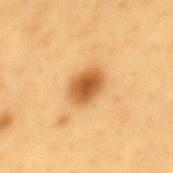Imaged during a routine full-body skin examination; the lesion was not biopsied and no histopathology is available.
A region of skin cropped from a whole-body photographic capture, roughly 15 mm wide.
About 3.5 mm across.
A male subject, aged around 60.
The lesion is located on the mid back.
This is a cross-polarized tile.